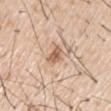The lesion was photographed on a routine skin check and not biopsied; there is no pathology result.
The patient is a male roughly 55 years of age.
Measured at roughly 3 mm in maximum diameter.
An algorithmic analysis of the crop reported an outline eccentricity of about 0.8 (0 = round, 1 = elongated) and two-axis asymmetry of about 0.35. The software also gave an average lesion color of about L≈61 a*≈19 b*≈32 (CIELAB) and a normalized border contrast of about 8. The analysis additionally found a border-irregularity index near 3.5/10, a color-variation rating of about 3/10, and peripheral color asymmetry of about 1.
A lesion tile, about 15 mm wide, cut from a 3D total-body photograph.
Captured under white-light illumination.